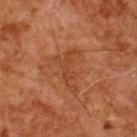workup — catalogued during a skin exam; not biopsied
image source — 15 mm crop, total-body photography
anatomic site — the upper back
patient — male, about 80 years old